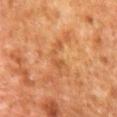Impression:
Recorded during total-body skin imaging; not selected for excision or biopsy.
Acquisition and patient details:
A male subject, in their mid-60s. This image is a 15 mm lesion crop taken from a total-body photograph. Captured under cross-polarized illumination. The lesion-visualizer software estimated an eccentricity of roughly 0.95 and a symmetry-axis asymmetry near 0.6. It also reported a border-irregularity rating of about 9.5/10 and a peripheral color-asymmetry measure near 0.5. Located on the back. About 5 mm across.